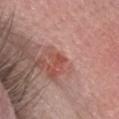biopsy status = imaged on a skin check; not biopsied | subject = male, aged 48–52 | body site = the head or neck | illumination = white-light | image source = 15 mm crop, total-body photography | lesion diameter = ≈1.5 mm.